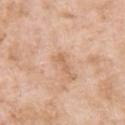illumination — white-light | image-analysis metrics — a lesion area of about 3 mm², an eccentricity of roughly 0.9, and a symmetry-axis asymmetry near 0.35; a lesion–skin lightness drop of about 7 and a normalized lesion–skin contrast near 5 | lesion diameter — ~3 mm (longest diameter) | site — the arm | patient — female, roughly 75 years of age | acquisition — ~15 mm tile from a whole-body skin photo.